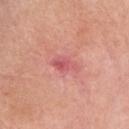The lesion was tiled from a total-body skin photograph and was not biopsied.
The patient is a female aged 63–67.
A close-up tile cropped from a whole-body skin photograph, about 15 mm across.
From the head or neck.
Measured at roughly 3 mm in maximum diameter.